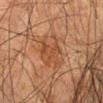Q: Is there a histopathology result?
A: no biopsy performed (imaged during a skin exam)
Q: Lesion location?
A: the right forearm
Q: Who is the patient?
A: male, in their 80s
Q: What lighting was used for the tile?
A: cross-polarized
Q: What kind of image is this?
A: total-body-photography crop, ~15 mm field of view